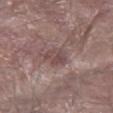This lesion was catalogued during total-body skin photography and was not selected for biopsy. The subject is a male aged around 80. The lesion is on the right forearm. A close-up tile cropped from a whole-body skin photograph, about 15 mm across.